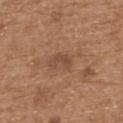| key | value |
|---|---|
| notes | no biopsy performed (imaged during a skin exam) |
| patient | male, aged approximately 70 |
| anatomic site | the back |
| illumination | white-light |
| diameter | about 3 mm |
| image | ~15 mm crop, total-body skin-cancer survey |